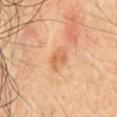No biopsy was performed on this lesion — it was imaged during a full skin examination and was not determined to be concerning. This image is a 15 mm lesion crop taken from a total-body photograph. A male patient, aged 63 to 67. Imaged with cross-polarized lighting. From the chest. An algorithmic analysis of the crop reported an area of roughly 4 mm², an eccentricity of roughly 0.8, and a shape-asymmetry score of about 0.45 (0 = symmetric). The analysis additionally found an average lesion color of about L≈63 a*≈25 b*≈40 (CIELAB), roughly 9 lightness units darker than nearby skin, and a normalized border contrast of about 6.5. And it measured a border-irregularity rating of about 4.5/10 and a within-lesion color-variation index near 1.5/10.